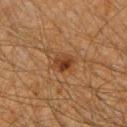notes: imaged on a skin check; not biopsied
imaging modality: ~15 mm crop, total-body skin-cancer survey
illumination: cross-polarized illumination
subject: male, approximately 35 years of age
body site: the right forearm